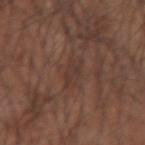Q: Was a biopsy performed?
A: total-body-photography surveillance lesion; no biopsy
Q: How was this image acquired?
A: ~15 mm tile from a whole-body skin photo
Q: What is the anatomic site?
A: the left forearm
Q: Who is the patient?
A: male, aged 63–67
Q: What lighting was used for the tile?
A: white-light illumination
Q: What is the lesion's diameter?
A: ≈3.5 mm